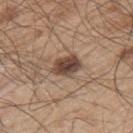<lesion>
  <biopsy_status>not biopsied; imaged during a skin examination</biopsy_status>
  <lesion_size>
    <long_diameter_mm_approx>3.5</long_diameter_mm_approx>
  </lesion_size>
  <patient>
    <sex>male</sex>
    <age_approx>50</age_approx>
  </patient>
  <site>back</site>
  <image>
    <source>total-body photography crop</source>
    <field_of_view_mm>15</field_of_view_mm>
  </image>
  <lighting>white-light</lighting>
</lesion>